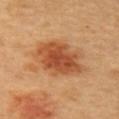On the back. The lesion's longest dimension is about 6.5 mm. A roughly 15 mm field-of-view crop from a total-body skin photograph. The tile uses cross-polarized illumination. A female patient, aged around 60.